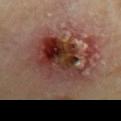Q: Is there a histopathology result?
A: no biopsy performed (imaged during a skin exam)
Q: What is the anatomic site?
A: the left upper arm
Q: What is the imaging modality?
A: ~15 mm crop, total-body skin-cancer survey
Q: What lighting was used for the tile?
A: cross-polarized
Q: What are the patient's age and sex?
A: male, about 70 years old